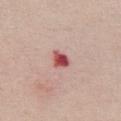<case>
  <biopsy_status>not biopsied; imaged during a skin examination</biopsy_status>
  <lighting>white-light</lighting>
  <image>
    <source>total-body photography crop</source>
    <field_of_view_mm>15</field_of_view_mm>
  </image>
  <patient>
    <sex>female</sex>
    <age_approx>55</age_approx>
  </patient>
  <site>chest</site>
</case>